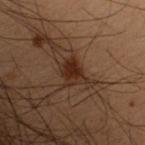  biopsy_status: not biopsied; imaged during a skin examination
  lesion_size:
    long_diameter_mm_approx: 3.0
  automated_metrics:
    area_mm2_approx: 6.5
    eccentricity: 0.65
    shape_asymmetry: 0.3
    cielab_L: 23
    cielab_a: 17
    cielab_b: 23
    vs_skin_contrast_norm: 9.5
    nevus_likeness_0_100: 85
    lesion_detection_confidence_0_100: 100
  lighting: cross-polarized
  patient:
    sex: female
    age_approx: 50
  site: arm
  image:
    source: total-body photography crop
    field_of_view_mm: 15